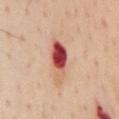follow-up=catalogued during a skin exam; not biopsied | diameter=~5.5 mm (longest diameter) | automated metrics=a lesion color around L≈53 a*≈32 b*≈29 in CIELAB, about 19 CIELAB-L* units darker than the surrounding skin, and a normalized lesion–skin contrast near 12; a classifier nevus-likeness of about 0/100 | patient=male, about 55 years old | anatomic site=the chest | imaging modality=~15 mm tile from a whole-body skin photo.